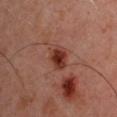| field | value |
|---|---|
| notes | total-body-photography surveillance lesion; no biopsy |
| location | the right upper arm |
| patient | male, aged around 45 |
| image | total-body-photography crop, ~15 mm field of view |